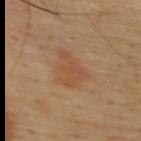Assessment:
Part of a total-body skin-imaging series; this lesion was reviewed on a skin check and was not flagged for biopsy.
Background:
Measured at roughly 4 mm in maximum diameter. The lesion is on the upper back. This image is a 15 mm lesion crop taken from a total-body photograph. The lesion-visualizer software estimated a color-variation rating of about 2/10. Imaged with cross-polarized lighting. A male subject, roughly 45 years of age.